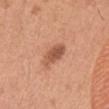Clinical impression:
This lesion was catalogued during total-body skin photography and was not selected for biopsy.
Context:
This is a white-light tile. On the left thigh. This image is a 15 mm lesion crop taken from a total-body photograph. A male patient aged 63–67.